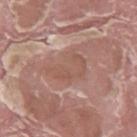<case>
  <patient>
    <sex>male</sex>
    <age_approx>40</age_approx>
  </patient>
  <image>
    <source>total-body photography crop</source>
    <field_of_view_mm>15</field_of_view_mm>
  </image>
  <site>left thigh</site>
  <lighting>white-light</lighting>
</case>